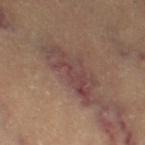notes=no biopsy performed (imaged during a skin exam)